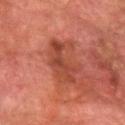image source: ~15 mm tile from a whole-body skin photo
body site: the left forearm
patient: male, in their 80s
lighting: cross-polarized illumination
image-analysis metrics: a lesion area of about 10 mm² and a symmetry-axis asymmetry near 0.45; a border-irregularity rating of about 6/10, internal color variation of about 2.5 on a 0–10 scale, and a peripheral color-asymmetry measure near 0.5